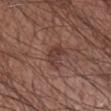notes: catalogued during a skin exam; not biopsied
location: the arm
subject: male, aged 28 to 32
illumination: white-light illumination
size: ≈3 mm
automated metrics: an area of roughly 6.5 mm², an outline eccentricity of about 0.65 (0 = round, 1 = elongated), and a shape-asymmetry score of about 0.4 (0 = symmetric)
image source: total-body-photography crop, ~15 mm field of view